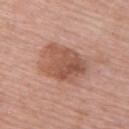| key | value |
|---|---|
| patient | female, in their mid- to late 60s |
| image | ~15 mm tile from a whole-body skin photo |
| body site | the upper back |
| automated lesion analysis | an eccentricity of roughly 0.75 and two-axis asymmetry of about 0.15; an average lesion color of about L≈54 a*≈23 b*≈29 (CIELAB), about 11 CIELAB-L* units darker than the surrounding skin, and a normalized border contrast of about 7.5; a border-irregularity index near 2.5/10, a color-variation rating of about 5.5/10, and peripheral color asymmetry of about 2; a classifier nevus-likeness of about 10/100 and a detector confidence of about 100 out of 100 that the crop contains a lesion |
| size | ~6 mm (longest diameter) |